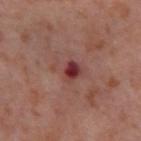Case summary:
– biopsy status · total-body-photography surveillance lesion; no biopsy
– location · the right thigh
– patient · female, aged 53 to 57
– lesion size · ≈3.5 mm
– imaging modality · ~15 mm tile from a whole-body skin photo
– tile lighting · cross-polarized illumination